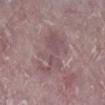follow-up: imaged on a skin check; not biopsied | lesion size: ≈6.5 mm | subject: female, approximately 65 years of age | site: the right lower leg | imaging modality: 15 mm crop, total-body photography.